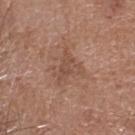Recorded during total-body skin imaging; not selected for excision or biopsy. Cropped from a total-body skin-imaging series; the visible field is about 15 mm. Located on the head or neck. An algorithmic analysis of the crop reported a lesion–skin lightness drop of about 7 and a normalized border contrast of about 5.5. The software also gave a border-irregularity rating of about 5.5/10. The patient is a female roughly 75 years of age. The recorded lesion diameter is about 3.5 mm. Imaged with white-light lighting.